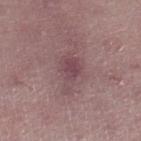Imaged during a routine full-body skin examination; the lesion was not biopsied and no histopathology is available. A female patient in their mid-40s. Measured at roughly 4.5 mm in maximum diameter. Located on the right lower leg. The tile uses white-light illumination. A 15 mm crop from a total-body photograph taken for skin-cancer surveillance. Automated image analysis of the tile measured an area of roughly 8 mm², an outline eccentricity of about 0.8 (0 = round, 1 = elongated), and a shape-asymmetry score of about 0.4 (0 = symmetric). It also reported a classifier nevus-likeness of about 0/100 and lesion-presence confidence of about 100/100.